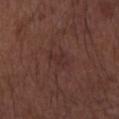Assessment:
Part of a total-body skin-imaging series; this lesion was reviewed on a skin check and was not flagged for biopsy.
Background:
A 15 mm crop from a total-body photograph taken for skin-cancer surveillance. A male patient aged around 75. The recorded lesion diameter is about 2.5 mm. Located on the arm. Captured under white-light illumination.